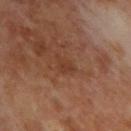workup = total-body-photography surveillance lesion; no biopsy
patient = male, aged around 70
location = the upper back
imaging modality = total-body-photography crop, ~15 mm field of view
illumination = cross-polarized illumination
lesion diameter = ≈2.5 mm